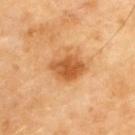| key | value |
|---|---|
| workup | imaged on a skin check; not biopsied |
| site | the upper back |
| imaging modality | total-body-photography crop, ~15 mm field of view |
| lesion size | ≈4 mm |
| automated metrics | an average lesion color of about L≈56 a*≈26 b*≈44 (CIELAB), about 13 CIELAB-L* units darker than the surrounding skin, and a normalized lesion–skin contrast near 8.5; border irregularity of about 2 on a 0–10 scale, internal color variation of about 3.5 on a 0–10 scale, and a peripheral color-asymmetry measure near 1; an automated nevus-likeness rating near 90 out of 100 and lesion-presence confidence of about 100/100 |
| patient | male, about 70 years old |
| tile lighting | cross-polarized |